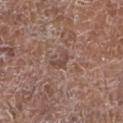Imaged during a routine full-body skin examination; the lesion was not biopsied and no histopathology is available. From the left lower leg. An algorithmic analysis of the crop reported a border-irregularity rating of about 7/10 and a within-lesion color-variation index near 0/10. Measured at roughly 2.5 mm in maximum diameter. Cropped from a total-body skin-imaging series; the visible field is about 15 mm. The patient is a female aged 78–82.